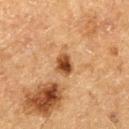| key | value |
|---|---|
| notes | no biopsy performed (imaged during a skin exam) |
| acquisition | total-body-photography crop, ~15 mm field of view |
| lesion diameter | ~2.5 mm (longest diameter) |
| subject | male, aged 73–77 |
| body site | the left thigh |